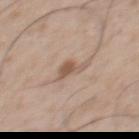Q: Was a biopsy performed?
A: no biopsy performed (imaged during a skin exam)
Q: What kind of image is this?
A: ~15 mm crop, total-body skin-cancer survey
Q: Who is the patient?
A: male, in their mid- to late 50s
Q: What is the anatomic site?
A: the upper back
Q: What did automated image analysis measure?
A: a classifier nevus-likeness of about 60/100 and a detector confidence of about 100 out of 100 that the crop contains a lesion
Q: Lesion size?
A: ≈3 mm